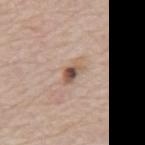The lesion was photographed on a routine skin check and not biopsied; there is no pathology result. The tile uses white-light illumination. Located on the mid back. A roughly 15 mm field-of-view crop from a total-body skin photograph. The recorded lesion diameter is about 3.5 mm. The lesion-visualizer software estimated a lesion area of about 5 mm², an eccentricity of roughly 0.85, and a symmetry-axis asymmetry near 0.35. It also reported an average lesion color of about L≈55 a*≈16 b*≈28 (CIELAB), roughly 12 lightness units darker than nearby skin, and a normalized border contrast of about 8.5. And it measured a border-irregularity index near 3.5/10, internal color variation of about 10 on a 0–10 scale, and a peripheral color-asymmetry measure near 3.5. And it measured an automated nevus-likeness rating near 75 out of 100. A male subject, aged 73–77.